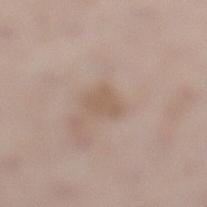{"patient": {"sex": "female", "age_approx": 40}, "lighting": "white-light", "site": "left lower leg", "image": {"source": "total-body photography crop", "field_of_view_mm": 15}, "lesion_size": {"long_diameter_mm_approx": 2.5}}